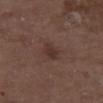* biopsy status — catalogued during a skin exam; not biopsied
* patient — male, in their mid- to late 70s
* acquisition — ~15 mm tile from a whole-body skin photo
* lighting — white-light illumination
* site — the right thigh
* lesion diameter — about 2.5 mm